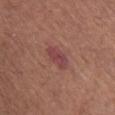Clinical impression: The lesion was tiled from a total-body skin photograph and was not biopsied. Background: The tile uses white-light illumination. The recorded lesion diameter is about 3.5 mm. The lesion-visualizer software estimated a shape eccentricity near 0.85 and a symmetry-axis asymmetry near 0.2. Located on the chest. A female subject aged 63–67. A 15 mm crop from a total-body photograph taken for skin-cancer surveillance.